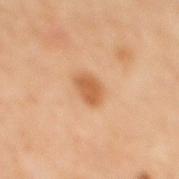A lesion tile, about 15 mm wide, cut from a 3D total-body photograph. The total-body-photography lesion software estimated a lesion color around L≈55 a*≈23 b*≈37 in CIELAB and a normalized border contrast of about 8. The analysis additionally found an automated nevus-likeness rating near 95 out of 100. A male patient, aged approximately 60. On the mid back. The recorded lesion diameter is about 3 mm. The tile uses cross-polarized illumination.